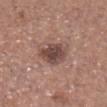Part of a total-body skin-imaging series; this lesion was reviewed on a skin check and was not flagged for biopsy. The patient is a male aged around 75. From the head or neck. A 15 mm close-up extracted from a 3D total-body photography capture.